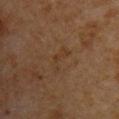Q: Is there a histopathology result?
A: total-body-photography surveillance lesion; no biopsy
Q: What did automated image analysis measure?
A: an area of roughly 4.5 mm², a shape eccentricity near 0.9, and a shape-asymmetry score of about 0.55 (0 = symmetric); a border-irregularity index near 6/10, internal color variation of about 0.5 on a 0–10 scale, and a peripheral color-asymmetry measure near 0
Q: How was the tile lit?
A: cross-polarized illumination
Q: Lesion size?
A: ≈3.5 mm
Q: What kind of image is this?
A: ~15 mm crop, total-body skin-cancer survey
Q: What is the anatomic site?
A: the left upper arm
Q: Patient demographics?
A: male, aged 58 to 62